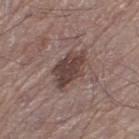Context: The lesion is located on the left thigh. A 15 mm crop from a total-body photograph taken for skin-cancer surveillance. Captured under white-light illumination. A male subject about 65 years old.